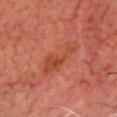The lesion was photographed on a routine skin check and not biopsied; there is no pathology result.
Located on the head or neck.
A 15 mm crop from a total-body photograph taken for skin-cancer surveillance.
A male subject in their 70s.
The tile uses cross-polarized illumination.
The lesion's longest dimension is about 4.5 mm.